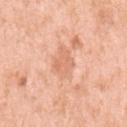{
  "biopsy_status": "not biopsied; imaged during a skin examination",
  "lesion_size": {
    "long_diameter_mm_approx": 3.5
  },
  "patient": {
    "sex": "female",
    "age_approx": 40
  },
  "image": {
    "source": "total-body photography crop",
    "field_of_view_mm": 15
  },
  "automated_metrics": {
    "area_mm2_approx": 7.5,
    "eccentricity": 0.65,
    "vs_skin_darker_L": 7.0,
    "vs_skin_contrast_norm": 4.5,
    "lesion_detection_confidence_0_100": 100
  },
  "site": "left upper arm"
}